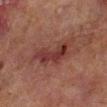Part of a total-body skin-imaging series; this lesion was reviewed on a skin check and was not flagged for biopsy. The lesion-visualizer software estimated a lesion color around L≈29 a*≈21 b*≈20 in CIELAB and a normalized border contrast of about 8.5. The software also gave peripheral color asymmetry of about 1.5. And it measured a detector confidence of about 100 out of 100 that the crop contains a lesion. A 15 mm crop from a total-body photograph taken for skin-cancer surveillance. A male subject roughly 70 years of age. About 6.5 mm across. The tile uses cross-polarized illumination. On the left lower leg.